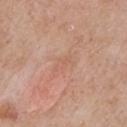Q: Is there a histopathology result?
A: total-body-photography surveillance lesion; no biopsy
Q: How large is the lesion?
A: ≈2.5 mm
Q: What kind of image is this?
A: ~15 mm tile from a whole-body skin photo
Q: Patient demographics?
A: female, roughly 60 years of age
Q: Illumination type?
A: white-light
Q: Where on the body is the lesion?
A: the chest